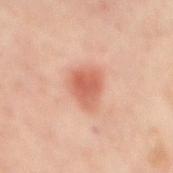| key | value |
|---|---|
| follow-up | catalogued during a skin exam; not biopsied |
| imaging modality | total-body-photography crop, ~15 mm field of view |
| patient | about 55 years old |
| illumination | cross-polarized illumination |
| automated lesion analysis | a lesion area of about 8.5 mm², an eccentricity of roughly 0.7, and a shape-asymmetry score of about 0.3 (0 = symmetric); a mean CIELAB color near L≈60 a*≈28 b*≈32, about 12 CIELAB-L* units darker than the surrounding skin, and a normalized lesion–skin contrast near 7.5; lesion-presence confidence of about 100/100 |
| lesion size | ~4 mm (longest diameter) |
| anatomic site | the back |